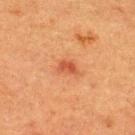Image and clinical context: The subject is a male approximately 40 years of age. The lesion is located on the back. A lesion tile, about 15 mm wide, cut from a 3D total-body photograph. Captured under cross-polarized illumination.